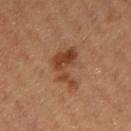{
  "biopsy_status": "not biopsied; imaged during a skin examination",
  "patient": {
    "sex": "female",
    "age_approx": 60
  },
  "image": {
    "source": "total-body photography crop",
    "field_of_view_mm": 15
  },
  "site": "left thigh"
}